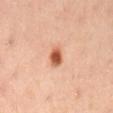Image and clinical context: Longest diameter approximately 2.5 mm. A 15 mm crop from a total-body photograph taken for skin-cancer surveillance. Located on the mid back. The tile uses cross-polarized illumination. The patient is a male roughly 55 years of age.